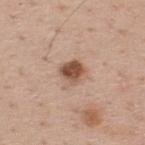notes = imaged on a skin check; not biopsied
subject = male, aged approximately 40
location = the upper back
image source = total-body-photography crop, ~15 mm field of view
lesion size = ~3 mm (longest diameter)